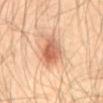{
  "biopsy_status": "not biopsied; imaged during a skin examination",
  "lighting": "cross-polarized",
  "patient": {
    "sex": "male",
    "age_approx": 55
  },
  "site": "abdomen",
  "image": {
    "source": "total-body photography crop",
    "field_of_view_mm": 15
  }
}